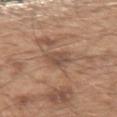workup = total-body-photography surveillance lesion; no biopsy
location = the left upper arm
subject = male, aged 48 to 52
size = about 3 mm
image = 15 mm crop, total-body photography